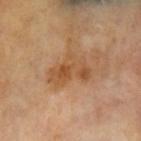Q: Is there a histopathology result?
A: imaged on a skin check; not biopsied
Q: What are the patient's age and sex?
A: male, in their mid- to late 60s
Q: Automated lesion metrics?
A: an eccentricity of roughly 0.75 and a symmetry-axis asymmetry near 0.5; lesion-presence confidence of about 100/100
Q: How was the tile lit?
A: cross-polarized illumination
Q: Where on the body is the lesion?
A: the left upper arm
Q: What is the lesion's diameter?
A: ~5 mm (longest diameter)
Q: What is the imaging modality?
A: ~15 mm crop, total-body skin-cancer survey